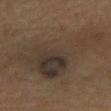The lesion was tiled from a total-body skin photograph and was not biopsied. An algorithmic analysis of the crop reported an area of roughly 44 mm² and a shape-asymmetry score of about 0.6 (0 = symmetric). It also reported a lesion color around L≈29 a*≈9 b*≈19 in CIELAB, a lesion–skin lightness drop of about 6, and a lesion-to-skin contrast of about 7 (normalized; higher = more distinct). It also reported a border-irregularity index near 8/10 and a within-lesion color-variation index near 6/10. It also reported an automated nevus-likeness rating near 25 out of 100. This is a cross-polarized tile. The patient is a female aged 38 to 42. The lesion is located on the left forearm. Cropped from a whole-body photographic skin survey; the tile spans about 15 mm.